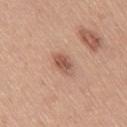No biopsy was performed on this lesion — it was imaged during a full skin examination and was not determined to be concerning. Approximately 3 mm at its widest. A 15 mm close-up tile from a total-body photography series done for melanoma screening. The subject is a female aged 53 to 57. This is a white-light tile. Located on the right thigh.